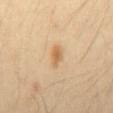Findings:
– workup: total-body-photography surveillance lesion; no biopsy
– subject: male, in their 40s
– tile lighting: cross-polarized illumination
– automated metrics: a lesion area of about 4 mm² and a shape-asymmetry score of about 0.2 (0 = symmetric); a mean CIELAB color near L≈64 a*≈19 b*≈40, a lesion–skin lightness drop of about 10, and a normalized lesion–skin contrast near 7; a nevus-likeness score of about 80/100 and a lesion-detection confidence of about 100/100
– lesion diameter: ~3 mm (longest diameter)
– image: ~15 mm tile from a whole-body skin photo
– location: the abdomen